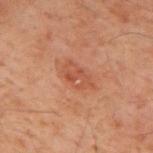Assessment: This lesion was catalogued during total-body skin photography and was not selected for biopsy. Acquisition and patient details: The subject is a male in their 60s. A 15 mm close-up tile from a total-body photography series done for melanoma screening. Located on the upper back. The tile uses cross-polarized illumination.